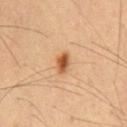Clinical impression:
Captured during whole-body skin photography for melanoma surveillance; the lesion was not biopsied.
Context:
The patient is a male about 60 years old. Measured at roughly 2.5 mm in maximum diameter. This is a cross-polarized tile. On the chest. A lesion tile, about 15 mm wide, cut from a 3D total-body photograph.